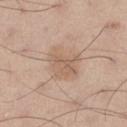biopsy status — no biopsy performed (imaged during a skin exam)
body site — the right thigh
subject — male, aged around 60
image source — 15 mm crop, total-body photography